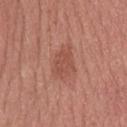acquisition = 15 mm crop, total-body photography | size = ≈4.5 mm | anatomic site = the upper back | illumination = white-light | patient = male, aged 23–27.